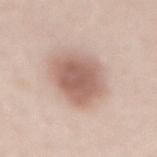Captured during whole-body skin photography for melanoma surveillance; the lesion was not biopsied.
Captured under white-light illumination.
A lesion tile, about 15 mm wide, cut from a 3D total-body photograph.
The lesion's longest dimension is about 5.5 mm.
The total-body-photography lesion software estimated an area of roughly 20 mm², an eccentricity of roughly 0.6, and a shape-asymmetry score of about 0.2 (0 = symmetric). It also reported a mean CIELAB color near L≈60 a*≈19 b*≈25, a lesion–skin lightness drop of about 14, and a lesion-to-skin contrast of about 8.5 (normalized; higher = more distinct).
A male patient, aged 63–67.
On the lower back.